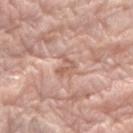The lesion was photographed on a routine skin check and not biopsied; there is no pathology result. A lesion tile, about 15 mm wide, cut from a 3D total-body photograph. Approximately 2.5 mm at its widest. A male subject approximately 80 years of age. On the right forearm.